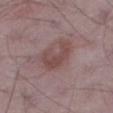Assessment: Recorded during total-body skin imaging; not selected for excision or biopsy. Clinical summary: The recorded lesion diameter is about 4.5 mm. A 15 mm close-up extracted from a 3D total-body photography capture. A male subject in their 50s. From the leg. The lesion-visualizer software estimated a lesion area of about 8.5 mm² and an outline eccentricity of about 0.85 (0 = round, 1 = elongated). This is a white-light tile.